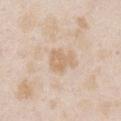Q: Is there a histopathology result?
A: imaged on a skin check; not biopsied